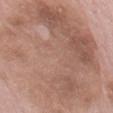biopsy status: total-body-photography surveillance lesion; no biopsy | subject: female, roughly 65 years of age | image-analysis metrics: an average lesion color of about L≈58 a*≈19 b*≈26 (CIELAB), a lesion–skin lightness drop of about 9, and a lesion-to-skin contrast of about 6.5 (normalized; higher = more distinct); a border-irregularity rating of about 4.5/10 and a within-lesion color-variation index near 6.5/10; a nevus-likeness score of about 5/100 and lesion-presence confidence of about 100/100 | size: about 20 mm | location: the mid back | imaging modality: 15 mm crop, total-body photography | illumination: white-light illumination.